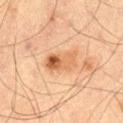<tbp_lesion>
  <biopsy_status>not biopsied; imaged during a skin examination</biopsy_status>
  <lesion_size>
    <long_diameter_mm_approx>4.5</long_diameter_mm_approx>
  </lesion_size>
  <image>
    <source>total-body photography crop</source>
    <field_of_view_mm>15</field_of_view_mm>
  </image>
  <site>left thigh</site>
  <patient>
    <sex>male</sex>
    <age_approx>70</age_approx>
  </patient>
</tbp_lesion>